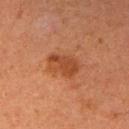Clinical impression:
Captured during whole-body skin photography for melanoma surveillance; the lesion was not biopsied.
Acquisition and patient details:
The lesion is on the right upper arm. A 15 mm close-up extracted from a 3D total-body photography capture. A female patient in their 60s. The recorded lesion diameter is about 4 mm.